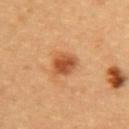Captured during whole-body skin photography for melanoma surveillance; the lesion was not biopsied. From the upper back. A 15 mm close-up tile from a total-body photography series done for melanoma screening. A female subject, roughly 30 years of age. Automated tile analysis of the lesion measured a classifier nevus-likeness of about 95/100 and a lesion-detection confidence of about 100/100. The tile uses cross-polarized illumination.